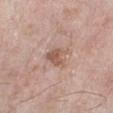notes: no biopsy performed (imaged during a skin exam) | illumination: white-light illumination | subject: female, aged 73–77 | imaging modality: ~15 mm crop, total-body skin-cancer survey | body site: the right lower leg.